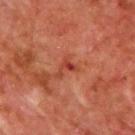| field | value |
|---|---|
| biopsy status | catalogued during a skin exam; not biopsied |
| size | ~3 mm (longest diameter) |
| image source | total-body-photography crop, ~15 mm field of view |
| subject | male, approximately 65 years of age |
| tile lighting | cross-polarized illumination |
| location | the upper back |